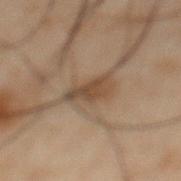The lesion was tiled from a total-body skin photograph and was not biopsied. The lesion is located on the abdomen. A region of skin cropped from a whole-body photographic capture, roughly 15 mm wide. The recorded lesion diameter is about 3.5 mm. Captured under cross-polarized illumination. The subject is a male roughly 50 years of age. Automated image analysis of the tile measured a lesion area of about 5.5 mm², an eccentricity of roughly 0.85, and a shape-asymmetry score of about 0.4 (0 = symmetric). It also reported a lesion color around L≈34 a*≈12 b*≈23 in CIELAB and roughly 7 lightness units darker than nearby skin. And it measured a detector confidence of about 100 out of 100 that the crop contains a lesion.